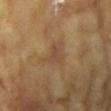biopsy status: total-body-photography surveillance lesion; no biopsy | tile lighting: cross-polarized illumination | automated lesion analysis: an area of roughly 6 mm², an outline eccentricity of about 0.5 (0 = round, 1 = elongated), and a shape-asymmetry score of about 0.45 (0 = symmetric); a mean CIELAB color near L≈47 a*≈16 b*≈32, roughly 6 lightness units darker than nearby skin, and a normalized lesion–skin contrast near 4.5; border irregularity of about 5.5 on a 0–10 scale, a within-lesion color-variation index near 2.5/10, and radial color variation of about 1; a classifier nevus-likeness of about 0/100 and a detector confidence of about 100 out of 100 that the crop contains a lesion | patient: female, in their mid- to late 70s | image: total-body-photography crop, ~15 mm field of view | location: the right upper arm | lesion size: ~3.5 mm (longest diameter).